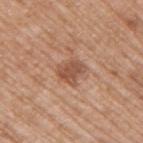{
  "site": "right upper arm",
  "image": {
    "source": "total-body photography crop",
    "field_of_view_mm": 15
  },
  "lesion_size": {
    "long_diameter_mm_approx": 3.0
  },
  "lighting": "white-light",
  "patient": {
    "sex": "male",
    "age_approx": 70
  }
}